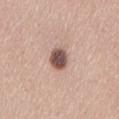Q: Was a biopsy performed?
A: imaged on a skin check; not biopsied
Q: What is the imaging modality?
A: ~15 mm crop, total-body skin-cancer survey
Q: Patient demographics?
A: female, aged around 30
Q: Lesion location?
A: the lower back